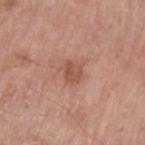Part of a total-body skin-imaging series; this lesion was reviewed on a skin check and was not flagged for biopsy.
The lesion-visualizer software estimated a footprint of about 4.5 mm², a shape eccentricity near 0.7, and two-axis asymmetry of about 0.3. And it measured a mean CIELAB color near L≈53 a*≈23 b*≈29 and a normalized border contrast of about 6.5. The analysis additionally found a border-irregularity index near 3/10. The software also gave a classifier nevus-likeness of about 5/100 and a detector confidence of about 100 out of 100 that the crop contains a lesion.
From the left upper arm.
The recorded lesion diameter is about 2.5 mm.
A region of skin cropped from a whole-body photographic capture, roughly 15 mm wide.
A male patient, aged 63 to 67.